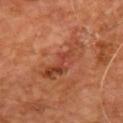notes: total-body-photography surveillance lesion; no biopsy | image-analysis metrics: a lesion area of about 12 mm², an eccentricity of roughly 0.95, and two-axis asymmetry of about 0.45; a mean CIELAB color near L≈40 a*≈26 b*≈32 and a normalized border contrast of about 6.5; a nevus-likeness score of about 0/100 and lesion-presence confidence of about 100/100 | size: about 7 mm | imaging modality: ~15 mm tile from a whole-body skin photo | patient: male, roughly 55 years of age | tile lighting: cross-polarized illumination | location: the chest.